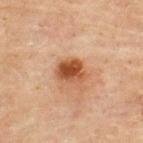Imaged during a routine full-body skin examination; the lesion was not biopsied and no histopathology is available.
A lesion tile, about 15 mm wide, cut from a 3D total-body photograph.
The lesion is on the upper back.
Approximately 3.5 mm at its widest.
A male patient about 45 years old.
Captured under cross-polarized illumination.